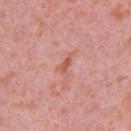Findings:
– notes · imaged on a skin check; not biopsied
– patient · female, roughly 40 years of age
– site · the right upper arm
– image · ~15 mm crop, total-body skin-cancer survey